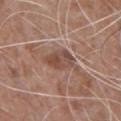Captured during whole-body skin photography for melanoma surveillance; the lesion was not biopsied. Captured under white-light illumination. Measured at roughly 4 mm in maximum diameter. Located on the upper back. The patient is a male aged approximately 60. Cropped from a whole-body photographic skin survey; the tile spans about 15 mm.